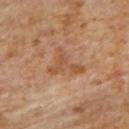Case summary:
- lighting · cross-polarized illumination
- automated metrics · border irregularity of about 8.5 on a 0–10 scale, internal color variation of about 2.5 on a 0–10 scale, and a peripheral color-asymmetry measure near 0.5
- subject · male, roughly 85 years of age or older
- body site · the upper back
- image source · ~15 mm tile from a whole-body skin photo
- lesion diameter · about 4 mm